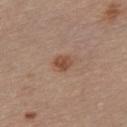follow-up = total-body-photography surveillance lesion; no biopsy
subject = female, approximately 65 years of age
anatomic site = the chest
size = ≈3 mm
acquisition = 15 mm crop, total-body photography
lighting = white-light